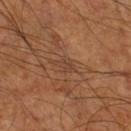Assessment:
The lesion was photographed on a routine skin check and not biopsied; there is no pathology result.
Image and clinical context:
Cropped from a total-body skin-imaging series; the visible field is about 15 mm. The lesion-visualizer software estimated a footprint of about 2.5 mm² and a shape-asymmetry score of about 0.3 (0 = symmetric). The analysis additionally found a lesion color around L≈40 a*≈20 b*≈29 in CIELAB and a normalized lesion–skin contrast near 5. It also reported a border-irregularity index near 3/10, a within-lesion color-variation index near 0/10, and radial color variation of about 0. It also reported an automated nevus-likeness rating near 0 out of 100 and lesion-presence confidence of about 70/100. A male subject, aged 58–62. Located on the right lower leg. The tile uses cross-polarized illumination. The recorded lesion diameter is about 2.5 mm.